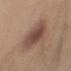Assessment:
Imaged during a routine full-body skin examination; the lesion was not biopsied and no histopathology is available.
Clinical summary:
A male subject approximately 50 years of age. Imaged with white-light lighting. Longest diameter approximately 5.5 mm. On the left upper arm. Automated tile analysis of the lesion measured an area of roughly 17 mm², a shape eccentricity near 0.75, and a shape-asymmetry score of about 0.15 (0 = symmetric). The software also gave a border-irregularity rating of about 2/10, a within-lesion color-variation index near 4/10, and a peripheral color-asymmetry measure near 1. It also reported a lesion-detection confidence of about 100/100. A lesion tile, about 15 mm wide, cut from a 3D total-body photograph.